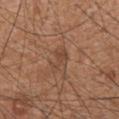Notes:
* follow-up — total-body-photography surveillance lesion; no biopsy
* image source — ~15 mm crop, total-body skin-cancer survey
* location — the abdomen
* patient — male, aged 63–67